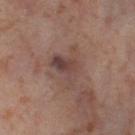Q: Was a biopsy performed?
A: no biopsy performed (imaged during a skin exam)
Q: Automated lesion metrics?
A: an eccentricity of roughly 0.7 and two-axis asymmetry of about 0.55; a classifier nevus-likeness of about 0/100 and a detector confidence of about 100 out of 100 that the crop contains a lesion
Q: What is the anatomic site?
A: the right thigh
Q: How large is the lesion?
A: ≈5 mm
Q: Patient demographics?
A: female, in their mid- to late 50s
Q: Illumination type?
A: cross-polarized
Q: What is the imaging modality?
A: total-body-photography crop, ~15 mm field of view